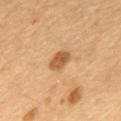Impression: The lesion was photographed on a routine skin check and not biopsied; there is no pathology result. Context: About 3 mm across. Cropped from a whole-body photographic skin survey; the tile spans about 15 mm. On the back. A male subject, in their mid- to late 50s.